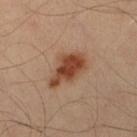This lesion was catalogued during total-body skin photography and was not selected for biopsy. The lesion-visualizer software estimated border irregularity of about 3.5 on a 0–10 scale, a color-variation rating of about 4.5/10, and peripheral color asymmetry of about 1.5. It also reported an automated nevus-likeness rating near 95 out of 100. A lesion tile, about 15 mm wide, cut from a 3D total-body photograph. Captured under cross-polarized illumination. A male subject in their 40s. The lesion is on the right lower leg. Measured at roughly 5.5 mm in maximum diameter.